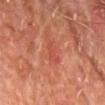Impression:
This lesion was catalogued during total-body skin photography and was not selected for biopsy.
Background:
A roughly 15 mm field-of-view crop from a total-body skin photograph. The subject is a male aged 63–67. The lesion is located on the chest.